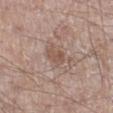Assessment:
No biopsy was performed on this lesion — it was imaged during a full skin examination and was not determined to be concerning.
Background:
The subject is a male aged approximately 60. A 15 mm crop from a total-body photograph taken for skin-cancer surveillance. The lesion is on the leg.